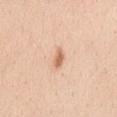Clinical impression:
Part of a total-body skin-imaging series; this lesion was reviewed on a skin check and was not flagged for biopsy.
Background:
The subject is a female about 25 years old. Automated image analysis of the tile measured a lesion area of about 3 mm², a shape eccentricity near 0.85, and a shape-asymmetry score of about 0.3 (0 = symmetric). And it measured an average lesion color of about L≈66 a*≈22 b*≈35 (CIELAB) and roughly 12 lightness units darker than nearby skin. The analysis additionally found internal color variation of about 1 on a 0–10 scale. The software also gave a nevus-likeness score of about 95/100 and a lesion-detection confidence of about 100/100. The lesion's longest dimension is about 2.5 mm. This image is a 15 mm lesion crop taken from a total-body photograph. Captured under white-light illumination. The lesion is located on the back.